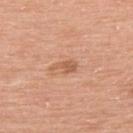| feature | finding |
|---|---|
| follow-up | catalogued during a skin exam; not biopsied |
| subject | male, roughly 60 years of age |
| illumination | white-light illumination |
| diameter | ~3 mm (longest diameter) |
| location | the back |
| imaging modality | 15 mm crop, total-body photography |